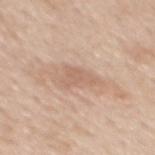Imaged during a routine full-body skin examination; the lesion was not biopsied and no histopathology is available.
An algorithmic analysis of the crop reported an area of roughly 4.5 mm². The software also gave a lesion color around L≈62 a*≈18 b*≈29 in CIELAB, roughly 8 lightness units darker than nearby skin, and a lesion-to-skin contrast of about 5 (normalized; higher = more distinct). The software also gave a peripheral color-asymmetry measure near 0.5.
From the mid back.
Approximately 3.5 mm at its widest.
A male subject, about 50 years old.
This image is a 15 mm lesion crop taken from a total-body photograph.
Captured under white-light illumination.